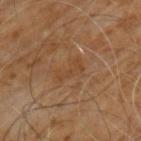Part of a total-body skin-imaging series; this lesion was reviewed on a skin check and was not flagged for biopsy.
A male subject, roughly 60 years of age.
The lesion is located on the chest.
The recorded lesion diameter is about 3.5 mm.
Captured under cross-polarized illumination.
Cropped from a whole-body photographic skin survey; the tile spans about 15 mm.
The lesion-visualizer software estimated a nevus-likeness score of about 0/100.